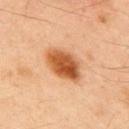Findings:
• lighting: cross-polarized illumination
• anatomic site: the upper back
• subject: male, roughly 50 years of age
• automated lesion analysis: a lesion area of about 12 mm² and an outline eccentricity of about 0.75 (0 = round, 1 = elongated); a border-irregularity rating of about 2/10 and peripheral color asymmetry of about 2.5
• imaging modality: 15 mm crop, total-body photography
• size: ~5 mm (longest diameter)